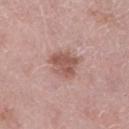{"lighting": "white-light", "image": {"source": "total-body photography crop", "field_of_view_mm": 15}, "site": "right lower leg", "automated_metrics": {"border_irregularity_0_10": 3.5, "color_variation_0_10": 3.0, "peripheral_color_asymmetry": 1.0}, "lesion_size": {"long_diameter_mm_approx": 3.5}, "patient": {"sex": "female", "age_approx": 40}}